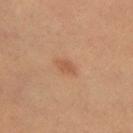Part of a total-body skin-imaging series; this lesion was reviewed on a skin check and was not flagged for biopsy.
A female patient, aged 28–32.
Automated image analysis of the tile measured an area of roughly 3 mm², a shape eccentricity near 0.75, and two-axis asymmetry of about 0.25. It also reported a nevus-likeness score of about 70/100 and a lesion-detection confidence of about 100/100.
The lesion is on the left thigh.
Cropped from a total-body skin-imaging series; the visible field is about 15 mm.
Longest diameter approximately 2.5 mm.
Captured under cross-polarized illumination.